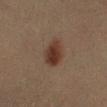{"biopsy_status": "not biopsied; imaged during a skin examination", "automated_metrics": {"border_irregularity_0_10": 2.0, "color_variation_0_10": 3.5, "peripheral_color_asymmetry": 1.0, "nevus_likeness_0_100": 100, "lesion_detection_confidence_0_100": 100}, "image": {"source": "total-body photography crop", "field_of_view_mm": 15}, "lighting": "cross-polarized", "patient": {"sex": "female", "age_approx": 60}, "site": "left lower leg", "lesion_size": {"long_diameter_mm_approx": 3.5}}